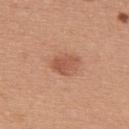The lesion was tiled from a total-body skin photograph and was not biopsied.
From the upper back.
Captured under white-light illumination.
A female patient in their mid- to late 30s.
Cropped from a total-body skin-imaging series; the visible field is about 15 mm.
Automated image analysis of the tile measured a footprint of about 5 mm², a shape eccentricity near 0.7, and a symmetry-axis asymmetry near 0.35. The analysis additionally found border irregularity of about 3.5 on a 0–10 scale and a peripheral color-asymmetry measure near 1.